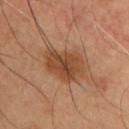Part of a total-body skin-imaging series; this lesion was reviewed on a skin check and was not flagged for biopsy. A male patient aged 53 to 57. The lesion is on the chest. A lesion tile, about 15 mm wide, cut from a 3D total-body photograph. Automated tile analysis of the lesion measured an area of roughly 14 mm², a shape eccentricity near 0.7, and a symmetry-axis asymmetry near 0.25. It also reported border irregularity of about 3 on a 0–10 scale, internal color variation of about 4 on a 0–10 scale, and a peripheral color-asymmetry measure near 1.5. Approximately 5.5 mm at its widest. Imaged with cross-polarized lighting.